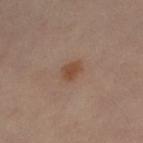Case summary:
- biopsy status: catalogued during a skin exam; not biopsied
- image-analysis metrics: a mean CIELAB color near L≈39 a*≈17 b*≈26 and about 7 CIELAB-L* units darker than the surrounding skin; a within-lesion color-variation index near 1.5/10 and radial color variation of about 0.5; a nevus-likeness score of about 90/100 and a detector confidence of about 100 out of 100 that the crop contains a lesion
- body site: the right leg
- subject: female, aged 58–62
- lighting: cross-polarized illumination
- acquisition: ~15 mm tile from a whole-body skin photo